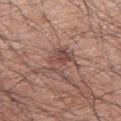Q: Was a biopsy performed?
A: catalogued during a skin exam; not biopsied
Q: Patient demographics?
A: male, aged around 70
Q: What is the lesion's diameter?
A: ~3.5 mm (longest diameter)
Q: How was this image acquired?
A: 15 mm crop, total-body photography
Q: Automated lesion metrics?
A: a lesion area of about 7.5 mm², an outline eccentricity of about 0.7 (0 = round, 1 = elongated), and two-axis asymmetry of about 0.4; an average lesion color of about L≈48 a*≈20 b*≈23 (CIELAB), a lesion–skin lightness drop of about 9, and a normalized lesion–skin contrast near 7; radial color variation of about 1.5; a nevus-likeness score of about 5/100
Q: What is the anatomic site?
A: the arm
Q: How was the tile lit?
A: white-light illumination